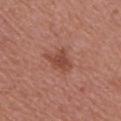Notes:
• biopsy status · catalogued during a skin exam; not biopsied
• automated lesion analysis · roughly 9 lightness units darker than nearby skin and a normalized lesion–skin contrast near 7; a border-irregularity rating of about 3.5/10 and radial color variation of about 0.5; lesion-presence confidence of about 100/100
• image source · ~15 mm crop, total-body skin-cancer survey
• diameter · ≈3.5 mm
• body site · the right upper arm
• subject · female, aged approximately 50